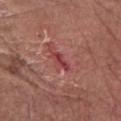Assessment: The lesion was tiled from a total-body skin photograph and was not biopsied. Background: Automated tile analysis of the lesion measured an area of roughly 3 mm², a shape eccentricity near 0.9, and two-axis asymmetry of about 0.3. The software also gave about 10 CIELAB-L* units darker than the surrounding skin and a lesion-to-skin contrast of about 7.5 (normalized; higher = more distinct). Longest diameter approximately 3 mm. From the right forearm. A region of skin cropped from a whole-body photographic capture, roughly 15 mm wide. This is a white-light tile. The subject is a male aged 73 to 77.